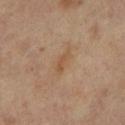The total-body-photography lesion software estimated a lesion area of about 2.5 mm² and two-axis asymmetry of about 0.3. The analysis additionally found a lesion color around L≈50 a*≈17 b*≈33 in CIELAB, roughly 6 lightness units darker than nearby skin, and a normalized lesion–skin contrast near 6. And it measured border irregularity of about 3 on a 0–10 scale and a peripheral color-asymmetry measure near 0. The software also gave an automated nevus-likeness rating near 0 out of 100 and a detector confidence of about 100 out of 100 that the crop contains a lesion. Measured at roughly 3 mm in maximum diameter. A 15 mm crop from a total-body photograph taken for skin-cancer surveillance. This is a cross-polarized tile. Located on the right leg. A female subject aged approximately 65.